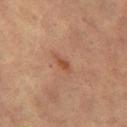Acquisition and patient details:
On the leg. The tile uses cross-polarized illumination. A female subject in their 70s. Cropped from a whole-body photographic skin survey; the tile spans about 15 mm.